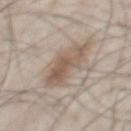image: total-body-photography crop, ~15 mm field of view
automated lesion analysis: an area of roughly 13 mm², an outline eccentricity of about 0.75 (0 = round, 1 = elongated), and two-axis asymmetry of about 0.3; about 9 CIELAB-L* units darker than the surrounding skin and a lesion-to-skin contrast of about 7 (normalized; higher = more distinct); border irregularity of about 4 on a 0–10 scale and internal color variation of about 4.5 on a 0–10 scale
subject: male, aged approximately 55
body site: the chest